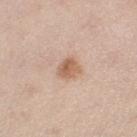<lesion>
  <biopsy_status>not biopsied; imaged during a skin examination</biopsy_status>
  <image>
    <source>total-body photography crop</source>
    <field_of_view_mm>15</field_of_view_mm>
  </image>
  <site>left thigh</site>
  <patient>
    <sex>female</sex>
    <age_approx>40</age_approx>
  </patient>
  <automated_metrics>
    <area_mm2_approx>5.0</area_mm2_approx>
    <shape_asymmetry>0.25</shape_asymmetry>
    <border_irregularity_0_10>2.0</border_irregularity_0_10>
    <color_variation_0_10>4.0</color_variation_0_10>
  </automated_metrics>
  <lighting>white-light</lighting>
</lesion>